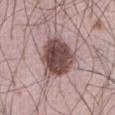Case summary:
• biopsy status: no biopsy performed (imaged during a skin exam)
• anatomic site: the abdomen
• acquisition: total-body-photography crop, ~15 mm field of view
• diameter: ≈6 mm
• lighting: white-light illumination
• subject: male, roughly 65 years of age